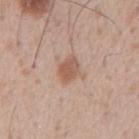{"biopsy_status": "not biopsied; imaged during a skin examination", "patient": {"sex": "male", "age_approx": 55}, "lesion_size": {"long_diameter_mm_approx": 3.0}, "automated_metrics": {"area_mm2_approx": 5.5, "shape_asymmetry": 0.2, "border_irregularity_0_10": 2.0, "peripheral_color_asymmetry": 1.0, "nevus_likeness_0_100": 45, "lesion_detection_confidence_0_100": 100}, "lighting": "white-light", "image": {"source": "total-body photography crop", "field_of_view_mm": 15}, "site": "abdomen"}